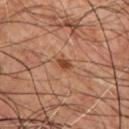{"biopsy_status": "not biopsied; imaged during a skin examination", "automated_metrics": {"area_mm2_approx": 2.5, "eccentricity": 0.75, "shape_asymmetry": 0.25, "border_irregularity_0_10": 2.5, "color_variation_0_10": 0.5}, "lighting": "cross-polarized", "patient": {"sex": "male", "age_approx": 55}, "image": {"source": "total-body photography crop", "field_of_view_mm": 15}, "lesion_size": {"long_diameter_mm_approx": 2.5}, "site": "front of the torso"}